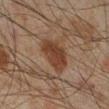Q: Was a biopsy performed?
A: imaged on a skin check; not biopsied
Q: What is the lesion's diameter?
A: ≈4.5 mm
Q: Patient demographics?
A: male, aged approximately 45
Q: Where on the body is the lesion?
A: the right lower leg
Q: Automated lesion metrics?
A: a lesion area of about 12 mm², an eccentricity of roughly 0.7, and a shape-asymmetry score of about 0.25 (0 = symmetric); a mean CIELAB color near L≈32 a*≈16 b*≈25, about 8 CIELAB-L* units darker than the surrounding skin, and a normalized border contrast of about 8.5; an automated nevus-likeness rating near 90 out of 100 and a detector confidence of about 100 out of 100 that the crop contains a lesion
Q: How was the tile lit?
A: cross-polarized illumination
Q: What is the imaging modality?
A: ~15 mm tile from a whole-body skin photo